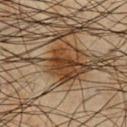Assessment: The lesion was tiled from a total-body skin photograph and was not biopsied.